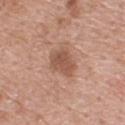Imaged during a routine full-body skin examination; the lesion was not biopsied and no histopathology is available. A male subject aged 53 to 57. Cropped from a whole-body photographic skin survey; the tile spans about 15 mm. An algorithmic analysis of the crop reported border irregularity of about 2.5 on a 0–10 scale, a within-lesion color-variation index near 2.5/10, and radial color variation of about 1. And it measured lesion-presence confidence of about 100/100. Longest diameter approximately 4 mm. On the upper back.